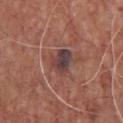notes = total-body-photography surveillance lesion; no biopsy | patient = male, in their mid- to late 60s | location = the chest | lesion diameter = about 3.5 mm | image source = ~15 mm crop, total-body skin-cancer survey | illumination = white-light illumination.